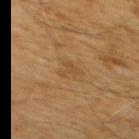Clinical impression:
The lesion was tiled from a total-body skin photograph and was not biopsied.
Clinical summary:
Cropped from a whole-body photographic skin survey; the tile spans about 15 mm. Located on the upper back. The subject is a male aged around 60. This is a cross-polarized tile.